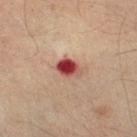Q: Was this lesion biopsied?
A: catalogued during a skin exam; not biopsied
Q: How large is the lesion?
A: about 3 mm
Q: Lesion location?
A: the right lower leg
Q: What kind of image is this?
A: ~15 mm tile from a whole-body skin photo
Q: Illumination type?
A: cross-polarized
Q: Automated lesion metrics?
A: an average lesion color of about L≈39 a*≈28 b*≈23 (CIELAB) and a lesion–skin lightness drop of about 17; a classifier nevus-likeness of about 0/100
Q: Patient demographics?
A: male, about 45 years old